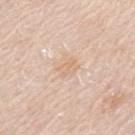imaging modality: ~15 mm tile from a whole-body skin photo; anatomic site: the left upper arm; illumination: white-light; subject: male, about 75 years old; lesion size: about 3 mm.